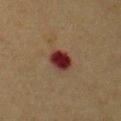Imaged during a routine full-body skin examination; the lesion was not biopsied and no histopathology is available.
Cropped from a whole-body photographic skin survey; the tile spans about 15 mm.
The patient is a male in their mid-50s.
The lesion is on the chest.